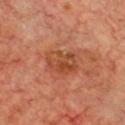The lesion was photographed on a routine skin check and not biopsied; there is no pathology result.
The lesion is located on the chest.
Measured at roughly 4.5 mm in maximum diameter.
The total-body-photography lesion software estimated a lesion area of about 10 mm² and two-axis asymmetry of about 0.2. The analysis additionally found border irregularity of about 2.5 on a 0–10 scale and internal color variation of about 6 on a 0–10 scale. And it measured a nevus-likeness score of about 0/100.
Captured under cross-polarized illumination.
A male patient, approximately 70 years of age.
Cropped from a whole-body photographic skin survey; the tile spans about 15 mm.